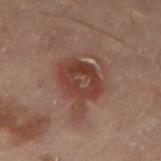The lesion was tiled from a total-body skin photograph and was not biopsied. A female patient, about 55 years old. The lesion is located on the lower back. The tile uses cross-polarized illumination. Cropped from a whole-body photographic skin survey; the tile spans about 15 mm. The lesion-visualizer software estimated a lesion area of about 14 mm², a shape eccentricity near 0.6, and a symmetry-axis asymmetry near 0.2. And it measured a mean CIELAB color near L≈32 a*≈19 b*≈21 and a lesion-to-skin contrast of about 8 (normalized; higher = more distinct). The lesion's longest dimension is about 4.5 mm.